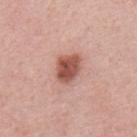{
  "biopsy_status": "not biopsied; imaged during a skin examination",
  "lighting": "white-light",
  "lesion_size": {
    "long_diameter_mm_approx": 4.0
  },
  "patient": {
    "sex": "male",
    "age_approx": 55
  },
  "image": {
    "source": "total-body photography crop",
    "field_of_view_mm": 15
  },
  "site": "mid back"
}